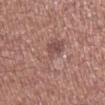workup — catalogued during a skin exam; not biopsied | image — 15 mm crop, total-body photography | site — the right lower leg | subject — male, aged around 70 | size — about 5.5 mm.